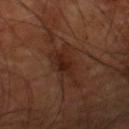workup = catalogued during a skin exam; not biopsied | lesion size = about 6 mm | imaging modality = ~15 mm crop, total-body skin-cancer survey | patient = male, aged 58–62 | tile lighting = cross-polarized illumination | anatomic site = the right lower leg.